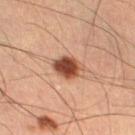Captured during whole-body skin photography for melanoma surveillance; the lesion was not biopsied. The lesion is located on the right lower leg. A roughly 15 mm field-of-view crop from a total-body skin photograph. The subject is a male aged approximately 55. Captured under cross-polarized illumination. An algorithmic analysis of the crop reported a footprint of about 7.5 mm². The analysis additionally found a lesion color around L≈41 a*≈21 b*≈28 in CIELAB, roughly 16 lightness units darker than nearby skin, and a normalized border contrast of about 12. The software also gave a nevus-likeness score of about 100/100 and a lesion-detection confidence of about 100/100. Longest diameter approximately 3.5 mm.